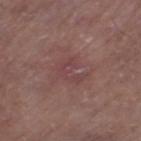No biopsy was performed on this lesion — it was imaged during a full skin examination and was not determined to be concerning.
A 15 mm close-up tile from a total-body photography series done for melanoma screening.
A male patient about 80 years old.
Located on the left thigh.